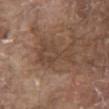workup: no biopsy performed (imaged during a skin exam)
patient: male, aged approximately 80
lesion size: ~6 mm (longest diameter)
image source: total-body-photography crop, ~15 mm field of view
location: the chest
automated metrics: a classifier nevus-likeness of about 0/100 and a lesion-detection confidence of about 75/100